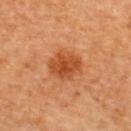The lesion was photographed on a routine skin check and not biopsied; there is no pathology result.
The lesion is on the upper back.
A female subject aged approximately 50.
This is a cross-polarized tile.
The total-body-photography lesion software estimated a lesion color around L≈49 a*≈30 b*≈42 in CIELAB, about 11 CIELAB-L* units darker than the surrounding skin, and a lesion-to-skin contrast of about 8 (normalized; higher = more distinct). The analysis additionally found a lesion-detection confidence of about 100/100.
This image is a 15 mm lesion crop taken from a total-body photograph.